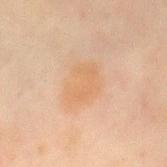This lesion was catalogued during total-body skin photography and was not selected for biopsy. Located on the chest. A female patient, about 50 years old. This image is a 15 mm lesion crop taken from a total-body photograph. An algorithmic analysis of the crop reported an area of roughly 11 mm², a shape eccentricity near 0.75, and a symmetry-axis asymmetry near 0.25. And it measured roughly 5 lightness units darker than nearby skin and a normalized lesion–skin contrast near 5.5. The lesion's longest dimension is about 5 mm. The tile uses cross-polarized illumination.